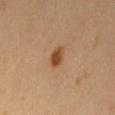| key | value |
|---|---|
| follow-up | catalogued during a skin exam; not biopsied |
| image source | total-body-photography crop, ~15 mm field of view |
| anatomic site | the leg |
| patient | male, about 50 years old |
| automated metrics | an average lesion color of about L≈47 a*≈22 b*≈36 (CIELAB), a lesion–skin lightness drop of about 12, and a normalized lesion–skin contrast near 9; border irregularity of about 2 on a 0–10 scale, a color-variation rating of about 3.5/10, and a peripheral color-asymmetry measure near 1.5; an automated nevus-likeness rating near 100 out of 100 and a detector confidence of about 100 out of 100 that the crop contains a lesion |